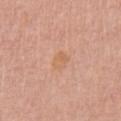Impression:
The lesion was tiled from a total-body skin photograph and was not biopsied.
Background:
The lesion is located on the chest. An algorithmic analysis of the crop reported an outline eccentricity of about 0.6 (0 = round, 1 = elongated) and a shape-asymmetry score of about 0.25 (0 = symmetric). This is a white-light tile. A lesion tile, about 15 mm wide, cut from a 3D total-body photograph. The patient is a female about 60 years old.